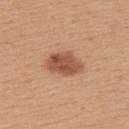Part of a total-body skin-imaging series; this lesion was reviewed on a skin check and was not flagged for biopsy. Approximately 4.5 mm at its widest. On the upper back. A female patient roughly 45 years of age. The tile uses white-light illumination. Cropped from a whole-body photographic skin survey; the tile spans about 15 mm.